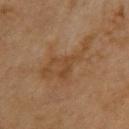workup: catalogued during a skin exam; not biopsied | patient: male, aged around 70 | image: 15 mm crop, total-body photography | size: ≈7 mm | site: the upper back | illumination: cross-polarized illumination | TBP lesion metrics: an automated nevus-likeness rating near 0 out of 100 and a detector confidence of about 100 out of 100 that the crop contains a lesion.